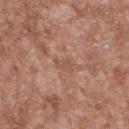Imaged during a routine full-body skin examination; the lesion was not biopsied and no histopathology is available.
Automated image analysis of the tile measured a footprint of about 3 mm², an eccentricity of roughly 0.8, and two-axis asymmetry of about 0.4. And it measured a lesion color around L≈53 a*≈22 b*≈30 in CIELAB, roughly 6 lightness units darker than nearby skin, and a lesion-to-skin contrast of about 5 (normalized; higher = more distinct). The software also gave a classifier nevus-likeness of about 0/100.
Measured at roughly 2.5 mm in maximum diameter.
The tile uses white-light illumination.
A lesion tile, about 15 mm wide, cut from a 3D total-body photograph.
The patient is a male in their mid-40s.
From the upper back.